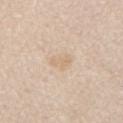Q: Was a biopsy performed?
A: imaged on a skin check; not biopsied
Q: How was this image acquired?
A: 15 mm crop, total-body photography
Q: How large is the lesion?
A: about 2.5 mm
Q: Automated lesion metrics?
A: a footprint of about 4.5 mm², an outline eccentricity of about 0.6 (0 = round, 1 = elongated), and a symmetry-axis asymmetry near 0.25; border irregularity of about 2.5 on a 0–10 scale and radial color variation of about 1
Q: What lighting was used for the tile?
A: white-light
Q: Where on the body is the lesion?
A: the mid back
Q: Who is the patient?
A: male, aged around 65